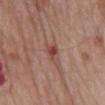The lesion was photographed on a routine skin check and not biopsied; there is no pathology result.
Cropped from a total-body skin-imaging series; the visible field is about 15 mm.
A male subject aged 78 to 82.
On the mid back.